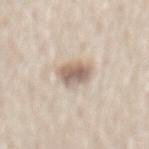Findings:
- biopsy status · no biopsy performed (imaged during a skin exam)
- location · the mid back
- image · ~15 mm crop, total-body skin-cancer survey
- patient · female, aged approximately 60